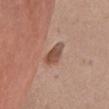Part of a total-body skin-imaging series; this lesion was reviewed on a skin check and was not flagged for biopsy.
From the chest.
A 15 mm crop from a total-body photograph taken for skin-cancer surveillance.
Captured under white-light illumination.
The lesion-visualizer software estimated an average lesion color of about L≈50 a*≈21 b*≈28 (CIELAB), a lesion–skin lightness drop of about 11, and a normalized lesion–skin contrast near 8.
A female patient roughly 65 years of age.
The lesion's longest dimension is about 4 mm.